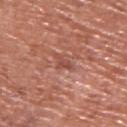No biopsy was performed on this lesion — it was imaged during a full skin examination and was not determined to be concerning.